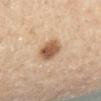Biopsy histopathology demonstrated a seborrheic keratosis.